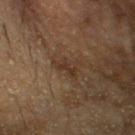Findings:
- follow-up: imaged on a skin check; not biopsied
- tile lighting: cross-polarized
- imaging modality: ~15 mm crop, total-body skin-cancer survey
- location: the head or neck
- patient: male, aged 83 or over
- lesion diameter: ~3.5 mm (longest diameter)